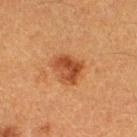patient:
  sex: female
  age_approx: 40
automated_metrics:
  area_mm2_approx: 9.5
  eccentricity: 0.55
  cielab_L: 40
  cielab_a: 23
  cielab_b: 33
  vs_skin_darker_L: 10.0
  vs_skin_contrast_norm: 8.0
image:
  source: total-body photography crop
  field_of_view_mm: 15
lighting: cross-polarized
site: right thigh
lesion_size:
  long_diameter_mm_approx: 3.5